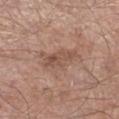<case>
  <biopsy_status>not biopsied; imaged during a skin examination</biopsy_status>
  <lesion_size>
    <long_diameter_mm_approx>4.5</long_diameter_mm_approx>
  </lesion_size>
  <automated_metrics>
    <area_mm2_approx>6.5</area_mm2_approx>
    <eccentricity>0.9</eccentricity>
    <shape_asymmetry>0.3</shape_asymmetry>
    <cielab_L>51</cielab_L>
    <cielab_a>20</cielab_a>
    <cielab_b>27</cielab_b>
    <vs_skin_darker_L>8.0</vs_skin_darker_L>
    <vs_skin_contrast_norm>6.0</vs_skin_contrast_norm>
  </automated_metrics>
  <lighting>white-light</lighting>
  <image>
    <source>total-body photography crop</source>
    <field_of_view_mm>15</field_of_view_mm>
  </image>
  <site>left lower leg</site>
  <patient>
    <sex>male</sex>
    <age_approx>55</age_approx>
  </patient>
</case>